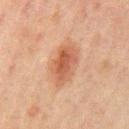Part of a total-body skin-imaging series; this lesion was reviewed on a skin check and was not flagged for biopsy. This is a cross-polarized tile. The recorded lesion diameter is about 5 mm. Located on the mid back. Cropped from a whole-body photographic skin survey; the tile spans about 15 mm. An algorithmic analysis of the crop reported a within-lesion color-variation index near 4.5/10 and radial color variation of about 1.5. The software also gave lesion-presence confidence of about 100/100. A male subject aged approximately 65.